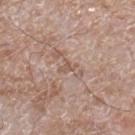Acquisition and patient details:
A 15 mm close-up extracted from a 3D total-body photography capture. The lesion-visualizer software estimated an average lesion color of about L≈56 a*≈18 b*≈26 (CIELAB) and a lesion-to-skin contrast of about 5.5 (normalized; higher = more distinct). The analysis additionally found an automated nevus-likeness rating near 0 out of 100 and a lesion-detection confidence of about 80/100. A male patient, aged 58 to 62. Approximately 2.5 mm at its widest. On the right lower leg.